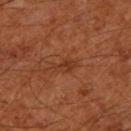The lesion was photographed on a routine skin check and not biopsied; there is no pathology result.
Imaged with cross-polarized lighting.
A male subject, roughly 65 years of age.
Cropped from a total-body skin-imaging series; the visible field is about 15 mm.
The lesion is on the left lower leg.
Longest diameter approximately 3 mm.
Automated image analysis of the tile measured a footprint of about 4 mm², an outline eccentricity of about 0.85 (0 = round, 1 = elongated), and a symmetry-axis asymmetry near 0.25. And it measured a lesion color around L≈34 a*≈24 b*≈31 in CIELAB. And it measured a border-irregularity index near 3/10 and radial color variation of about 0.5. The software also gave an automated nevus-likeness rating near 0 out of 100 and a lesion-detection confidence of about 100/100.